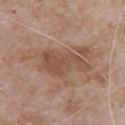The lesion was tiled from a total-body skin photograph and was not biopsied.
The patient is a male roughly 80 years of age.
The lesion is located on the front of the torso.
This image is a 15 mm lesion crop taken from a total-body photograph.